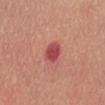Captured during whole-body skin photography for melanoma surveillance; the lesion was not biopsied. The lesion is located on the back. This image is a 15 mm lesion crop taken from a total-body photograph. A female subject about 65 years old.